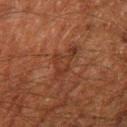Q: Was a biopsy performed?
A: total-body-photography surveillance lesion; no biopsy
Q: What is the anatomic site?
A: the leg
Q: Patient demographics?
A: male, aged approximately 60
Q: What is the imaging modality?
A: ~15 mm tile from a whole-body skin photo
Q: What did automated image analysis measure?
A: a lesion color around L≈27 a*≈19 b*≈25 in CIELAB, a lesion–skin lightness drop of about 5, and a lesion-to-skin contrast of about 5.5 (normalized; higher = more distinct); a border-irregularity index near 6.5/10, a color-variation rating of about 2/10, and a peripheral color-asymmetry measure near 0.5